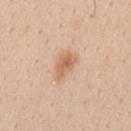Notes:
– workup — imaged on a skin check; not biopsied
– automated lesion analysis — an area of roughly 6.5 mm², an eccentricity of roughly 0.85, and a shape-asymmetry score of about 0.25 (0 = symmetric); a normalized border contrast of about 6.5; a classifier nevus-likeness of about 75/100
– patient — male, roughly 30 years of age
– image source — total-body-photography crop, ~15 mm field of view
– body site — the back